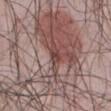biopsy status = imaged on a skin check; not biopsied | body site = the abdomen | patient = male, roughly 50 years of age | lesion diameter = about 1.5 mm | imaging modality = 15 mm crop, total-body photography.